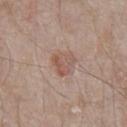{"biopsy_status": "not biopsied; imaged during a skin examination", "automated_metrics": {"cielab_L": 54, "cielab_a": 19, "cielab_b": 25, "vs_skin_darker_L": 8.0, "vs_skin_contrast_norm": 6.0, "nevus_likeness_0_100": 50, "lesion_detection_confidence_0_100": 100}, "lesion_size": {"long_diameter_mm_approx": 3.5}, "site": "chest", "image": {"source": "total-body photography crop", "field_of_view_mm": 15}, "patient": {"sex": "male", "age_approx": 65}, "lighting": "white-light"}